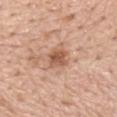Part of a total-body skin-imaging series; this lesion was reviewed on a skin check and was not flagged for biopsy.
The lesion is located on the mid back.
Longest diameter approximately 2.5 mm.
A 15 mm close-up extracted from a 3D total-body photography capture.
A male subject aged around 60.
An algorithmic analysis of the crop reported a footprint of about 5.5 mm², an outline eccentricity of about 0.5 (0 = round, 1 = elongated), and a symmetry-axis asymmetry near 0.25. The analysis additionally found a lesion color around L≈56 a*≈22 b*≈32 in CIELAB and a lesion–skin lightness drop of about 12. It also reported radial color variation of about 1.5. And it measured a classifier nevus-likeness of about 80/100 and lesion-presence confidence of about 100/100.